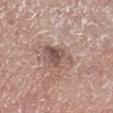This lesion was catalogued during total-body skin photography and was not selected for biopsy.
Cropped from a whole-body photographic skin survey; the tile spans about 15 mm.
The lesion is on the right lower leg.
The patient is a male roughly 75 years of age.
Measured at roughly 4 mm in maximum diameter.
This is a white-light tile.